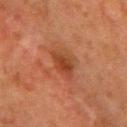Impression:
Recorded during total-body skin imaging; not selected for excision or biopsy.